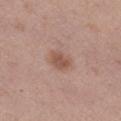workup: imaged on a skin check; not biopsied | patient: female, approximately 30 years of age | location: the right lower leg | tile lighting: white-light illumination | image-analysis metrics: a lesion area of about 5.5 mm² and a shape-asymmetry score of about 0.3 (0 = symmetric); a lesion–skin lightness drop of about 9 and a normalized lesion–skin contrast near 7; a detector confidence of about 100 out of 100 that the crop contains a lesion | imaging modality: 15 mm crop, total-body photography.